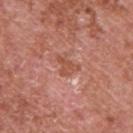{
  "biopsy_status": "not biopsied; imaged during a skin examination",
  "site": "back",
  "image": {
    "source": "total-body photography crop",
    "field_of_view_mm": 15
  },
  "patient": {
    "sex": "male",
    "age_approx": 70
  },
  "automated_metrics": {
    "area_mm2_approx": 3.5,
    "eccentricity": 0.8,
    "shape_asymmetry": 0.6,
    "cielab_L": 52,
    "cielab_a": 27,
    "cielab_b": 31,
    "vs_skin_darker_L": 8.0,
    "vs_skin_contrast_norm": 6.5,
    "border_irregularity_0_10": 6.0,
    "peripheral_color_asymmetry": 0.5
  },
  "lighting": "white-light",
  "lesion_size": {
    "long_diameter_mm_approx": 3.0
  }
}